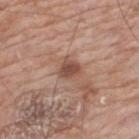Assessment:
Part of a total-body skin-imaging series; this lesion was reviewed on a skin check and was not flagged for biopsy.
Acquisition and patient details:
A male subject aged approximately 60. A 15 mm close-up extracted from a 3D total-body photography capture. On the back.